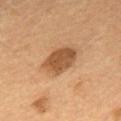<case>
  <biopsy_status>not biopsied; imaged during a skin examination</biopsy_status>
  <patient>
    <sex>male</sex>
    <age_approx>55</age_approx>
  </patient>
  <site>upper back</site>
  <lighting>cross-polarized</lighting>
  <image>
    <source>total-body photography crop</source>
    <field_of_view_mm>15</field_of_view_mm>
  </image>
</case>